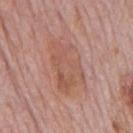workup: no biopsy performed (imaged during a skin exam)
automated lesion analysis: an area of roughly 19 mm² and a shape eccentricity near 0.8; a border-irregularity rating of about 3.5/10, a within-lesion color-variation index near 4/10, and peripheral color asymmetry of about 1.5
subject: male, aged around 75
imaging modality: ~15 mm tile from a whole-body skin photo
body site: the back
lighting: white-light illumination
diameter: ~6.5 mm (longest diameter)